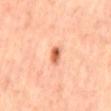Captured during whole-body skin photography for melanoma surveillance; the lesion was not biopsied.
Cropped from a whole-body photographic skin survey; the tile spans about 15 mm.
This is a cross-polarized tile.
On the mid back.
The subject is a male roughly 55 years of age.
Automated image analysis of the tile measured a shape eccentricity near 0.85 and a shape-asymmetry score of about 0.25 (0 = symmetric). And it measured about 16 CIELAB-L* units darker than the surrounding skin and a normalized border contrast of about 9. The analysis additionally found a lesion-detection confidence of about 100/100.
Longest diameter approximately 2.5 mm.